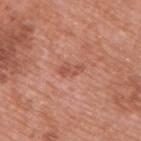Q: Is there a histopathology result?
A: imaged on a skin check; not biopsied
Q: What is the anatomic site?
A: the upper back
Q: What did automated image analysis measure?
A: an average lesion color of about L≈53 a*≈27 b*≈30 (CIELAB), a lesion–skin lightness drop of about 8, and a normalized lesion–skin contrast near 5.5; a classifier nevus-likeness of about 0/100 and a detector confidence of about 100 out of 100 that the crop contains a lesion
Q: How was the tile lit?
A: white-light
Q: What kind of image is this?
A: 15 mm crop, total-body photography
Q: What are the patient's age and sex?
A: male, aged around 70
Q: Lesion size?
A: ≈2.5 mm